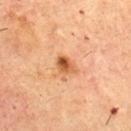follow-up: catalogued during a skin exam; not biopsied